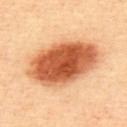A male patient roughly 40 years of age. Automated image analysis of the tile measured border irregularity of about 1.5 on a 0–10 scale, internal color variation of about 8 on a 0–10 scale, and radial color variation of about 2.5. The analysis additionally found a lesion-detection confidence of about 100/100. Approximately 9 mm at its widest. Imaged with cross-polarized lighting. The lesion is on the upper back. A roughly 15 mm field-of-view crop from a total-body skin photograph.